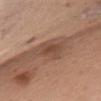The lesion was tiled from a total-body skin photograph and was not biopsied. The patient is a female about 55 years old. The lesion is on the chest. Imaged with white-light lighting. The lesion-visualizer software estimated an area of roughly 8 mm², an eccentricity of roughly 0.85, and a shape-asymmetry score of about 0.35 (0 = symmetric). The analysis additionally found a border-irregularity rating of about 4/10, a within-lesion color-variation index near 3/10, and peripheral color asymmetry of about 1. Measured at roughly 4.5 mm in maximum diameter. A 15 mm crop from a total-body photograph taken for skin-cancer surveillance.